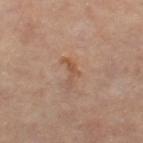Findings:
– biopsy status — total-body-photography surveillance lesion; no biopsy
– illumination — cross-polarized
– TBP lesion metrics — an area of roughly 3 mm², a shape eccentricity near 0.9, and a shape-asymmetry score of about 0.65 (0 = symmetric); a lesion color around L≈53 a*≈21 b*≈31 in CIELAB and roughly 8 lightness units darker than nearby skin; a within-lesion color-variation index near 0/10 and a peripheral color-asymmetry measure near 0; an automated nevus-likeness rating near 0 out of 100
– location — the left thigh
– subject — female, aged approximately 60
– image — ~15 mm crop, total-body skin-cancer survey